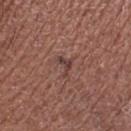workup = imaged on a skin check; not biopsied
imaging modality = ~15 mm crop, total-body skin-cancer survey
automated metrics = roughly 8 lightness units darker than nearby skin and a normalized lesion–skin contrast near 7; a nevus-likeness score of about 0/100 and a lesion-detection confidence of about 90/100
subject = female, aged around 50
location = the right lower leg
tile lighting = white-light
diameter = about 2.5 mm